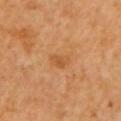Impression: Imaged during a routine full-body skin examination; the lesion was not biopsied and no histopathology is available. Acquisition and patient details: A region of skin cropped from a whole-body photographic capture, roughly 15 mm wide. A female subject in their mid-50s. From the left upper arm. The lesion's longest dimension is about 2.5 mm. Captured under cross-polarized illumination.